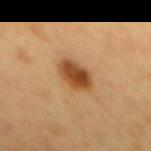follow-up = total-body-photography surveillance lesion; no biopsy | subject = female, approximately 50 years of age | site = the mid back | diameter = ≈4 mm | lighting = cross-polarized | acquisition = 15 mm crop, total-body photography.